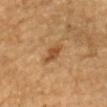Findings:
– biopsy status — total-body-photography surveillance lesion; no biopsy
– site — the head or neck
– patient — male, in their mid- to late 80s or older
– image — ~15 mm crop, total-body skin-cancer survey
– size — ≈3.5 mm
– automated metrics — a border-irregularity rating of about 2.5/10, a within-lesion color-variation index near 3/10, and a peripheral color-asymmetry measure near 1; a classifier nevus-likeness of about 70/100 and a detector confidence of about 100 out of 100 that the crop contains a lesion
– tile lighting — cross-polarized illumination